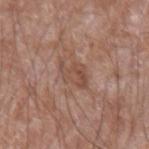Q: Is there a histopathology result?
A: imaged on a skin check; not biopsied
Q: Lesion location?
A: the left forearm
Q: Patient demographics?
A: male, about 60 years old
Q: How was this image acquired?
A: ~15 mm tile from a whole-body skin photo
Q: How was the tile lit?
A: white-light illumination